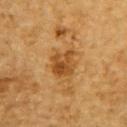The lesion was tiled from a total-body skin photograph and was not biopsied.
This image is a 15 mm lesion crop taken from a total-body photograph.
About 3.5 mm across.
Captured under cross-polarized illumination.
On the upper back.
A male patient, in their mid-80s or older.